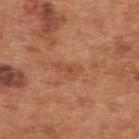The lesion was photographed on a routine skin check and not biopsied; there is no pathology result. The patient is a male aged approximately 65. Imaged with white-light lighting. This image is a 15 mm lesion crop taken from a total-body photograph. The lesion is on the upper back. Automated image analysis of the tile measured an eccentricity of roughly 0.9 and two-axis asymmetry of about 0.4. The analysis additionally found an average lesion color of about L≈50 a*≈25 b*≈34 (CIELAB), a lesion–skin lightness drop of about 7, and a lesion-to-skin contrast of about 5 (normalized; higher = more distinct). It also reported a border-irregularity rating of about 4.5/10, a within-lesion color-variation index near 0.5/10, and peripheral color asymmetry of about 0. And it measured a nevus-likeness score of about 0/100 and lesion-presence confidence of about 100/100.